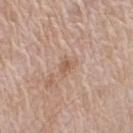The lesion was tiled from a total-body skin photograph and was not biopsied. A male patient approximately 65 years of age. Imaged with white-light lighting. Measured at roughly 2.5 mm in maximum diameter. Cropped from a whole-body photographic skin survey; the tile spans about 15 mm. The lesion is on the arm.